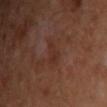Part of a total-body skin-imaging series; this lesion was reviewed on a skin check and was not flagged for biopsy. About 3 mm across. Captured under cross-polarized illumination. Located on the left arm. Automated tile analysis of the lesion measured a footprint of about 4 mm², an outline eccentricity of about 0.8 (0 = round, 1 = elongated), and a shape-asymmetry score of about 0.5 (0 = symmetric). It also reported a nevus-likeness score of about 0/100 and a lesion-detection confidence of about 100/100. A 15 mm close-up tile from a total-body photography series done for melanoma screening. A male subject, approximately 50 years of age.